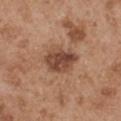{"lesion_size": {"long_diameter_mm_approx": 4.0}, "patient": {"sex": "male", "age_approx": 55}, "lighting": "white-light", "site": "left upper arm", "automated_metrics": {"area_mm2_approx": 10.0, "eccentricity": 0.65, "shape_asymmetry": 0.25, "nevus_likeness_0_100": 5}, "image": {"source": "total-body photography crop", "field_of_view_mm": 15}}